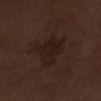Q: What is the lesion's diameter?
A: ≈5 mm
Q: How was the tile lit?
A: white-light
Q: What is the anatomic site?
A: the right lower leg
Q: Who is the patient?
A: male, aged 68–72
Q: What is the imaging modality?
A: 15 mm crop, total-body photography